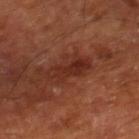Impression: Captured during whole-body skin photography for melanoma surveillance; the lesion was not biopsied. Context: Measured at roughly 4.5 mm in maximum diameter. This image is a 15 mm lesion crop taken from a total-body photograph. On the leg. The subject is approximately 65 years of age. An algorithmic analysis of the crop reported a border-irregularity index near 3.5/10, a within-lesion color-variation index near 4/10, and a peripheral color-asymmetry measure near 1.5. The software also gave a lesion-detection confidence of about 100/100.